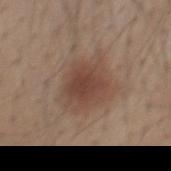No biopsy was performed on this lesion — it was imaged during a full skin examination and was not determined to be concerning. Imaged with white-light lighting. On the mid back. Automated image analysis of the tile measured a lesion color around L≈44 a*≈18 b*≈25 in CIELAB, a lesion–skin lightness drop of about 9, and a lesion-to-skin contrast of about 7.5 (normalized; higher = more distinct). The software also gave border irregularity of about 2.5 on a 0–10 scale, internal color variation of about 3 on a 0–10 scale, and a peripheral color-asymmetry measure near 1. The software also gave a nevus-likeness score of about 90/100 and a lesion-detection confidence of about 100/100. Measured at roughly 5 mm in maximum diameter. A male patient, aged 38–42. A roughly 15 mm field-of-view crop from a total-body skin photograph.